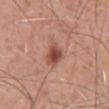The lesion was tiled from a total-body skin photograph and was not biopsied. A 15 mm crop from a total-body photograph taken for skin-cancer surveillance. The recorded lesion diameter is about 3 mm. A male patient aged 48 to 52. The total-body-photography lesion software estimated a lesion area of about 6 mm², a shape eccentricity near 0.5, and two-axis asymmetry of about 0.2. And it measured about 12 CIELAB-L* units darker than the surrounding skin and a normalized border contrast of about 8.5. And it measured a border-irregularity index near 2/10, a within-lesion color-variation index near 5/10, and peripheral color asymmetry of about 1.5. It also reported a nevus-likeness score of about 95/100 and a detector confidence of about 100 out of 100 that the crop contains a lesion. The tile uses white-light illumination. On the abdomen.